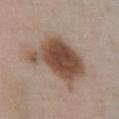{"lighting": "white-light", "patient": {"sex": "male", "age_approx": 55}, "image": {"source": "total-body photography crop", "field_of_view_mm": 15}, "site": "chest", "lesion_size": {"long_diameter_mm_approx": 7.5}, "automated_metrics": {"vs_skin_darker_L": 15.0, "vs_skin_contrast_norm": 11.0, "border_irregularity_0_10": 4.0, "color_variation_0_10": 5.0, "peripheral_color_asymmetry": 1.5, "lesion_detection_confidence_0_100": 100}}